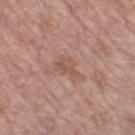Part of a total-body skin-imaging series; this lesion was reviewed on a skin check and was not flagged for biopsy.
The lesion is on the leg.
A lesion tile, about 15 mm wide, cut from a 3D total-body photograph.
Measured at roughly 4 mm in maximum diameter.
Automated image analysis of the tile measured an average lesion color of about L≈54 a*≈22 b*≈26 (CIELAB), a lesion–skin lightness drop of about 7, and a normalized lesion–skin contrast near 5.5. The analysis additionally found a border-irregularity rating of about 5/10, a within-lesion color-variation index near 2/10, and peripheral color asymmetry of about 1.
A female patient aged 58–62.
This is a white-light tile.